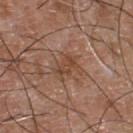Q: Was this lesion biopsied?
A: no biopsy performed (imaged during a skin exam)
Q: How was this image acquired?
A: 15 mm crop, total-body photography
Q: Lesion size?
A: ≈3 mm
Q: Who is the patient?
A: male, in their mid-50s
Q: What is the anatomic site?
A: the chest
Q: What did automated image analysis measure?
A: a lesion area of about 4 mm², an outline eccentricity of about 0.75 (0 = round, 1 = elongated), and a shape-asymmetry score of about 0.35 (0 = symmetric); a border-irregularity index near 4/10 and radial color variation of about 0.5; a classifier nevus-likeness of about 0/100